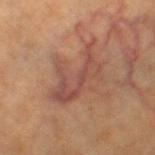<tbp_lesion>
  <image>
    <source>total-body photography crop</source>
    <field_of_view_mm>15</field_of_view_mm>
  </image>
  <lesion_size>
    <long_diameter_mm_approx>6.5</long_diameter_mm_approx>
  </lesion_size>
  <patient>
    <sex>female</sex>
    <age_approx>40</age_approx>
  </patient>
  <automated_metrics>
    <area_mm2_approx>18.0</area_mm2_approx>
    <eccentricity>0.75</eccentricity>
    <shape_asymmetry>0.5</shape_asymmetry>
    <vs_skin_contrast_norm>6.5</vs_skin_contrast_norm>
    <border_irregularity_0_10>7.5</border_irregularity_0_10>
    <color_variation_0_10>4.0</color_variation_0_10>
    <peripheral_color_asymmetry>1.5</peripheral_color_asymmetry>
  </automated_metrics>
  <lighting>cross-polarized</lighting>
  <site>right thigh</site>
</tbp_lesion>